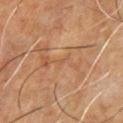Imaged during a routine full-body skin examination; the lesion was not biopsied and no histopathology is available. The lesion is on the chest. A region of skin cropped from a whole-body photographic capture, roughly 15 mm wide. Imaged with cross-polarized lighting. A male patient, roughly 60 years of age.